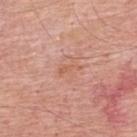Q: Was this lesion biopsied?
A: total-body-photography surveillance lesion; no biopsy
Q: What did automated image analysis measure?
A: an area of roughly 2 mm², an eccentricity of roughly 0.95, and a symmetry-axis asymmetry near 0.4; an average lesion color of about L≈60 a*≈24 b*≈32 (CIELAB), roughly 6 lightness units darker than nearby skin, and a normalized border contrast of about 5.5; a classifier nevus-likeness of about 0/100
Q: How was the tile lit?
A: white-light illumination
Q: Lesion size?
A: ≈3 mm
Q: What is the imaging modality?
A: 15 mm crop, total-body photography
Q: Lesion location?
A: the upper back
Q: Who is the patient?
A: male, aged approximately 60